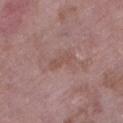The lesion was tiled from a total-body skin photograph and was not biopsied. The patient is a female in their 70s. The lesion is on the left lower leg. The lesion's longest dimension is about 3.5 mm. The tile uses white-light illumination. A 15 mm close-up tile from a total-body photography series done for melanoma screening.